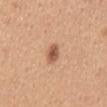Case summary:
- biopsy status · total-body-photography surveillance lesion; no biopsy
- patient · female, aged around 30
- anatomic site · the mid back
- imaging modality · total-body-photography crop, ~15 mm field of view